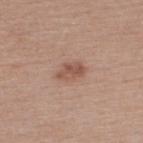Clinical impression:
This lesion was catalogued during total-body skin photography and was not selected for biopsy.
Background:
A lesion tile, about 15 mm wide, cut from a 3D total-body photograph. A male subject aged 38–42. On the upper back.